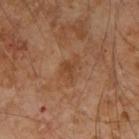Clinical impression: The lesion was tiled from a total-body skin photograph and was not biopsied. Image and clinical context: From the right upper arm. A 15 mm close-up tile from a total-body photography series done for melanoma screening. A male patient approximately 55 years of age.